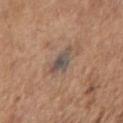workup: no biopsy performed (imaged during a skin exam) | automated lesion analysis: a lesion area of about 6 mm², an outline eccentricity of about 0.75 (0 = round, 1 = elongated), and a symmetry-axis asymmetry near 0.35; a mean CIELAB color near L≈49 a*≈12 b*≈21, about 9 CIELAB-L* units darker than the surrounding skin, and a normalized lesion–skin contrast near 9; border irregularity of about 3.5 on a 0–10 scale, a color-variation rating of about 7/10, and radial color variation of about 2; lesion-presence confidence of about 100/100 | size: about 3.5 mm | lighting: white-light illumination | anatomic site: the right upper arm | acquisition: total-body-photography crop, ~15 mm field of view | patient: female, aged around 65.